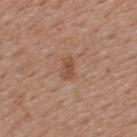• follow-up — total-body-photography surveillance lesion; no biopsy
• image — total-body-photography crop, ~15 mm field of view
• body site — the upper back
• size — ~2.5 mm (longest diameter)
• patient — male, about 55 years old
• illumination — white-light illumination